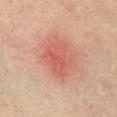Q: Where on the body is the lesion?
A: the chest
Q: What are the patient's age and sex?
A: female, aged approximately 65
Q: What lighting was used for the tile?
A: cross-polarized illumination
Q: What is the imaging modality?
A: ~15 mm tile from a whole-body skin photo